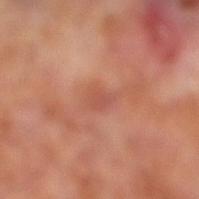The lesion was tiled from a total-body skin photograph and was not biopsied.
Captured under cross-polarized illumination.
A male patient, approximately 70 years of age.
The total-body-photography lesion software estimated an outline eccentricity of about 0.7 (0 = round, 1 = elongated). And it measured a mean CIELAB color near L≈51 a*≈27 b*≈30, roughly 7 lightness units darker than nearby skin, and a lesion-to-skin contrast of about 4.5 (normalized; higher = more distinct). It also reported a border-irregularity rating of about 2.5/10, a color-variation rating of about 1.5/10, and peripheral color asymmetry of about 0.5. It also reported a nevus-likeness score of about 0/100 and a lesion-detection confidence of about 100/100.
A close-up tile cropped from a whole-body skin photograph, about 15 mm across.
Measured at roughly 2.5 mm in maximum diameter.
From the right lower leg.